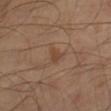workup — total-body-photography surveillance lesion; no biopsy
acquisition — 15 mm crop, total-body photography
body site — the leg
patient — male, in their mid-40s